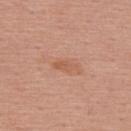biopsy_status: not biopsied; imaged during a skin examination
image:
  source: total-body photography crop
  field_of_view_mm: 15
lesion_size:
  long_diameter_mm_approx: 3.0
site: upper back
patient:
  sex: female
  age_approx: 55
lighting: white-light